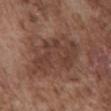Part of a total-body skin-imaging series; this lesion was reviewed on a skin check and was not flagged for biopsy.
This image is a 15 mm lesion crop taken from a total-body photograph.
The lesion is located on the front of the torso.
Longest diameter approximately 8 mm.
A male subject, aged 73–77.